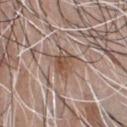notes: no biopsy performed (imaged during a skin exam); acquisition: ~15 mm tile from a whole-body skin photo; body site: the chest; patient: male, aged 43 to 47.